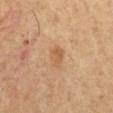{
  "biopsy_status": "not biopsied; imaged during a skin examination",
  "lighting": "cross-polarized",
  "patient": {
    "sex": "male",
    "age_approx": 60
  },
  "image": {
    "source": "total-body photography crop",
    "field_of_view_mm": 15
  },
  "site": "chest",
  "lesion_size": {
    "long_diameter_mm_approx": 3.0
  }
}